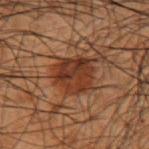The lesion was tiled from a total-body skin photograph and was not biopsied. The patient is a male aged 48–52. Measured at roughly 4.5 mm in maximum diameter. The lesion-visualizer software estimated a lesion area of about 14 mm² and a shape eccentricity near 0.5. The analysis additionally found an average lesion color of about L≈26 a*≈19 b*≈25 (CIELAB), a lesion–skin lightness drop of about 8, and a lesion-to-skin contrast of about 9 (normalized; higher = more distinct). It also reported a border-irregularity rating of about 2.5/10, internal color variation of about 4.5 on a 0–10 scale, and a peripheral color-asymmetry measure near 1.5. This image is a 15 mm lesion crop taken from a total-body photograph. Located on the left forearm.